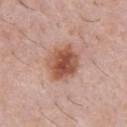workup: catalogued during a skin exam; not biopsied
size: ~4.5 mm (longest diameter)
location: the chest
acquisition: total-body-photography crop, ~15 mm field of view
illumination: white-light
patient: male, aged 48–52